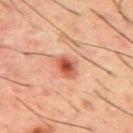patient — male, aged approximately 50; image — ~15 mm tile from a whole-body skin photo; illumination — cross-polarized; site — the mid back; diameter — ~3 mm (longest diameter).